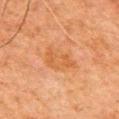No biopsy was performed on this lesion — it was imaged during a full skin examination and was not determined to be concerning. From the chest. A male patient aged 78 to 82. A 15 mm close-up extracted from a 3D total-body photography capture. The lesion's longest dimension is about 4 mm.